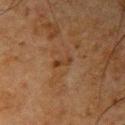Findings:
- automated lesion analysis: a nevus-likeness score of about 0/100 and a lesion-detection confidence of about 100/100
- tile lighting: cross-polarized
- diameter: ~2.5 mm (longest diameter)
- acquisition: total-body-photography crop, ~15 mm field of view
- body site: the arm
- patient: male, in their mid-60s